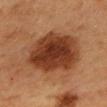{"biopsy_status": "not biopsied; imaged during a skin examination", "automated_metrics": {"nevus_likeness_0_100": 95, "lesion_detection_confidence_0_100": 100}, "lesion_size": {"long_diameter_mm_approx": 7.0}, "patient": {"sex": "female", "age_approx": 60}, "site": "mid back", "lighting": "cross-polarized", "image": {"source": "total-body photography crop", "field_of_view_mm": 15}}